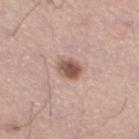notes: catalogued during a skin exam; not biopsied | subject: male, aged 33 to 37 | TBP lesion metrics: a lesion area of about 5 mm², a shape eccentricity near 0.7, and two-axis asymmetry of about 0.25; an automated nevus-likeness rating near 95 out of 100 and lesion-presence confidence of about 100/100 | anatomic site: the leg | lesion diameter: ≈3 mm | imaging modality: ~15 mm crop, total-body skin-cancer survey.